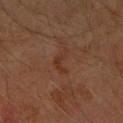workup: catalogued during a skin exam; not biopsied | lighting: cross-polarized illumination | imaging modality: ~15 mm tile from a whole-body skin photo | automated metrics: an outline eccentricity of about 0.85 (0 = round, 1 = elongated) and two-axis asymmetry of about 0.65; a border-irregularity index near 7/10 and internal color variation of about 0 on a 0–10 scale; a nevus-likeness score of about 0/100 and a detector confidence of about 100 out of 100 that the crop contains a lesion | site: the right forearm | subject: female, aged approximately 70.